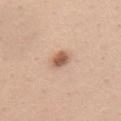subject=female, aged around 25; acquisition=~15 mm tile from a whole-body skin photo; site=the chest; tile lighting=white-light.